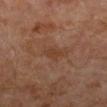{"biopsy_status": "not biopsied; imaged during a skin examination", "lighting": "cross-polarized", "automated_metrics": {"nevus_likeness_0_100": 0, "lesion_detection_confidence_0_100": 100}, "patient": {"sex": "male", "age_approx": 30}, "site": "left leg", "lesion_size": {"long_diameter_mm_approx": 2.5}, "image": {"source": "total-body photography crop", "field_of_view_mm": 15}}